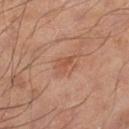follow-up = catalogued during a skin exam; not biopsied
lesion diameter = ~2.5 mm (longest diameter)
lighting = cross-polarized illumination
subject = male, roughly 55 years of age
body site = the left thigh
imaging modality = total-body-photography crop, ~15 mm field of view
automated lesion analysis = an area of roughly 3 mm², a shape eccentricity near 0.75, and a symmetry-axis asymmetry near 0.45; a border-irregularity index near 4/10, a within-lesion color-variation index near 1/10, and peripheral color asymmetry of about 0.5